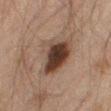Q: Is there a histopathology result?
A: catalogued during a skin exam; not biopsied
Q: Where on the body is the lesion?
A: the right thigh
Q: Who is the patient?
A: male, aged 58 to 62
Q: What did automated image analysis measure?
A: an area of roughly 17 mm² and a shape eccentricity near 0.7; an average lesion color of about L≈30 a*≈13 b*≈20 (CIELAB), a lesion–skin lightness drop of about 12, and a lesion-to-skin contrast of about 11.5 (normalized; higher = more distinct); an automated nevus-likeness rating near 95 out of 100 and a detector confidence of about 100 out of 100 that the crop contains a lesion
Q: What kind of image is this?
A: total-body-photography crop, ~15 mm field of view
Q: How was the tile lit?
A: cross-polarized illumination
Q: How large is the lesion?
A: ≈5 mm